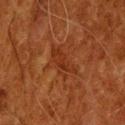Part of a total-body skin-imaging series; this lesion was reviewed on a skin check and was not flagged for biopsy. The subject is a male approximately 80 years of age. On the head or neck. Cropped from a total-body skin-imaging series; the visible field is about 15 mm. Captured under cross-polarized illumination.